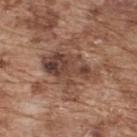Notes:
- biopsy status · total-body-photography surveillance lesion; no biopsy
- tile lighting · white-light illumination
- location · the upper back
- subject · male, approximately 75 years of age
- imaging modality · ~15 mm crop, total-body skin-cancer survey
- size · ≈6.5 mm
- TBP lesion metrics · an average lesion color of about L≈44 a*≈19 b*≈26 (CIELAB) and a lesion–skin lightness drop of about 11; internal color variation of about 7.5 on a 0–10 scale; an automated nevus-likeness rating near 0 out of 100 and a detector confidence of about 100 out of 100 that the crop contains a lesion